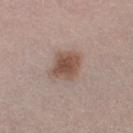Captured during whole-body skin photography for melanoma surveillance; the lesion was not biopsied.
This is a white-light tile.
The lesion is located on the leg.
A female patient, about 50 years old.
This image is a 15 mm lesion crop taken from a total-body photograph.
Automated tile analysis of the lesion measured a lesion area of about 10 mm² and a shape eccentricity near 0.5. The analysis additionally found a within-lesion color-variation index near 3.5/10 and peripheral color asymmetry of about 0.5. And it measured lesion-presence confidence of about 100/100.
Longest diameter approximately 3.5 mm.